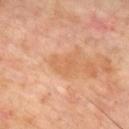body site=the front of the torso; imaging modality=~15 mm crop, total-body skin-cancer survey; patient=male, approximately 55 years of age.